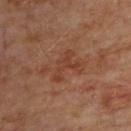Findings:
– biopsy status · imaged on a skin check; not biopsied
– illumination · cross-polarized
– lesion diameter · ≈4 mm
– subject · male, approximately 70 years of age
– anatomic site · the upper back
– image · ~15 mm tile from a whole-body skin photo